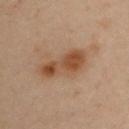{"biopsy_status": "not biopsied; imaged during a skin examination", "site": "right upper arm", "patient": {"sex": "male", "age_approx": 55}, "image": {"source": "total-body photography crop", "field_of_view_mm": 15}}